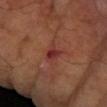biopsy status = no biopsy performed (imaged during a skin exam) | image source = ~15 mm tile from a whole-body skin photo | anatomic site = the left forearm | subject = male, in their mid- to late 50s | lighting = cross-polarized | size = ~2.5 mm (longest diameter).